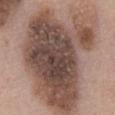Assessment: This lesion was catalogued during total-body skin photography and was not selected for biopsy. Background: From the chest. The subject is a female aged around 60. A roughly 15 mm field-of-view crop from a total-body skin photograph.